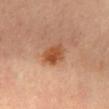This lesion was catalogued during total-body skin photography and was not selected for biopsy. The lesion is on the mid back. This is a cross-polarized tile. A male subject, about 55 years old. A close-up tile cropped from a whole-body skin photograph, about 15 mm across.